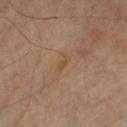notes: total-body-photography surveillance lesion; no biopsy | patient: male, aged 63–67 | lesion size: ~2.5 mm (longest diameter) | body site: the right upper arm | lighting: cross-polarized illumination | imaging modality: ~15 mm crop, total-body skin-cancer survey.